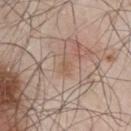Findings:
• notes: total-body-photography surveillance lesion; no biopsy
• tile lighting: white-light
• anatomic site: the chest
• imaging modality: 15 mm crop, total-body photography
• diameter: ~2.5 mm (longest diameter)
• subject: male, aged approximately 55